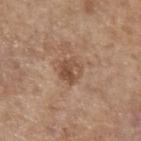{
  "biopsy_status": "not biopsied; imaged during a skin examination",
  "patient": {
    "sex": "female",
    "age_approx": 65
  },
  "automated_metrics": {
    "area_mm2_approx": 6.5,
    "eccentricity": 0.4,
    "shape_asymmetry": 0.25,
    "cielab_L": 50,
    "cielab_a": 19,
    "cielab_b": 30,
    "vs_skin_darker_L": 9.0,
    "vs_skin_contrast_norm": 7.0,
    "nevus_likeness_0_100": 0,
    "lesion_detection_confidence_0_100": 100
  },
  "site": "left forearm",
  "lesion_size": {
    "long_diameter_mm_approx": 3.0
  },
  "lighting": "white-light",
  "image": {
    "source": "total-body photography crop",
    "field_of_view_mm": 15
  }
}